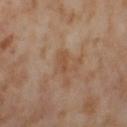This lesion was catalogued during total-body skin photography and was not selected for biopsy.
Imaged with cross-polarized lighting.
A close-up tile cropped from a whole-body skin photograph, about 15 mm across.
On the right thigh.
A female subject aged around 55.